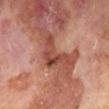{
  "biopsy_status": "not biopsied; imaged during a skin examination",
  "automated_metrics": {
    "area_mm2_approx": 9.0,
    "eccentricity": 0.85,
    "shape_asymmetry": 0.7,
    "cielab_L": 45,
    "cielab_a": 26,
    "cielab_b": 29,
    "vs_skin_contrast_norm": 8.0,
    "color_variation_0_10": 5.0,
    "peripheral_color_asymmetry": 1.5
  },
  "lesion_size": {
    "long_diameter_mm_approx": 5.5
  },
  "image": {
    "source": "total-body photography crop",
    "field_of_view_mm": 15
  },
  "site": "left lower leg",
  "patient": {
    "sex": "male",
    "age_approx": 70
  },
  "lighting": "cross-polarized"
}